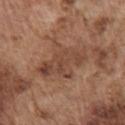Impression: Imaged during a routine full-body skin examination; the lesion was not biopsied and no histopathology is available. Context: Longest diameter approximately 6 mm. The lesion is located on the chest. A roughly 15 mm field-of-view crop from a total-body skin photograph. The subject is a male aged around 75. Automated tile analysis of the lesion measured a lesion area of about 16 mm², a shape eccentricity near 0.75, and two-axis asymmetry of about 0.3. The analysis additionally found a mean CIELAB color near L≈45 a*≈20 b*≈28, a lesion–skin lightness drop of about 8, and a normalized lesion–skin contrast near 6.5. The analysis additionally found a nevus-likeness score of about 0/100. The tile uses white-light illumination.